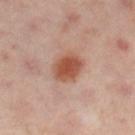notes: total-body-photography surveillance lesion; no biopsy
subject: female, in their 50s
anatomic site: the left lower leg
acquisition: total-body-photography crop, ~15 mm field of view
tile lighting: cross-polarized illumination